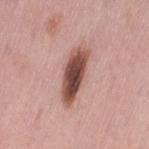Q: Was this lesion biopsied?
A: total-body-photography surveillance lesion; no biopsy
Q: Patient demographics?
A: female, about 50 years old
Q: Where on the body is the lesion?
A: the left thigh
Q: Illumination type?
A: white-light illumination
Q: What kind of image is this?
A: total-body-photography crop, ~15 mm field of view
Q: Lesion size?
A: ~6.5 mm (longest diameter)
Q: What did automated image analysis measure?
A: a lesion area of about 12 mm², a shape eccentricity near 0.95, and a symmetry-axis asymmetry near 0.2; a border-irregularity index near 3/10 and a color-variation rating of about 6.5/10